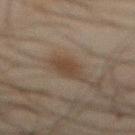Notes:
- workup: imaged on a skin check; not biopsied
- imaging modality: 15 mm crop, total-body photography
- location: the abdomen
- subject: male, roughly 45 years of age